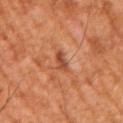<case>
<biopsy_status>not biopsied; imaged during a skin examination</biopsy_status>
<image>
  <source>total-body photography crop</source>
  <field_of_view_mm>15</field_of_view_mm>
</image>
<site>arm</site>
<patient>
  <sex>male</sex>
  <age_approx>65</age_approx>
</patient>
<automated_metrics>
  <eccentricity>0.9</eccentricity>
  <shape_asymmetry>0.25</shape_asymmetry>
  <border_irregularity_0_10>3.0</border_irregularity_0_10>
  <color_variation_0_10>0.0</color_variation_0_10>
  <peripheral_color_asymmetry>0.0</peripheral_color_asymmetry>
  <nevus_likeness_0_100>0</nevus_likeness_0_100>
  <lesion_detection_confidence_0_100>80</lesion_detection_confidence_0_100>
</automated_metrics>
<lighting>cross-polarized</lighting>
<lesion_size>
  <long_diameter_mm_approx>3.0</long_diameter_mm_approx>
</lesion_size>
</case>